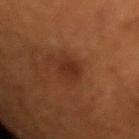Imaged during a routine full-body skin examination; the lesion was not biopsied and no histopathology is available. The total-body-photography lesion software estimated a lesion area of about 3.5 mm² and an outline eccentricity of about 0.75 (0 = round, 1 = elongated). And it measured a nevus-likeness score of about 15/100 and lesion-presence confidence of about 100/100. Captured under cross-polarized illumination. A lesion tile, about 15 mm wide, cut from a 3D total-body photograph. The patient is a female approximately 80 years of age. The lesion is on the right upper arm. The lesion's longest dimension is about 2.5 mm.